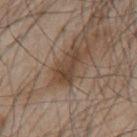Impression: The lesion was tiled from a total-body skin photograph and was not biopsied. Image and clinical context: The lesion is located on the mid back. The recorded lesion diameter is about 4 mm. The total-body-photography lesion software estimated an area of roughly 6 mm². And it measured a classifier nevus-likeness of about 20/100 and a detector confidence of about 100 out of 100 that the crop contains a lesion. The subject is a male roughly 45 years of age. Imaged with white-light lighting. Cropped from a total-body skin-imaging series; the visible field is about 15 mm.